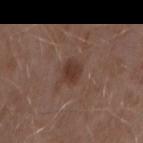The lesion was photographed on a routine skin check and not biopsied; there is no pathology result. A male patient, roughly 55 years of age. Located on the right upper arm. A 15 mm crop from a total-body photograph taken for skin-cancer surveillance.